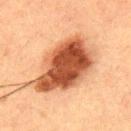workup: no biopsy performed (imaged during a skin exam)
illumination: cross-polarized
lesion size: about 9.5 mm
subject: male, aged 48–52
image: ~15 mm crop, total-body skin-cancer survey
image-analysis metrics: an area of roughly 35 mm²; a lesion color around L≈43 a*≈23 b*≈31 in CIELAB
site: the upper back